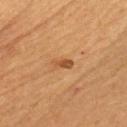The lesion was photographed on a routine skin check and not biopsied; there is no pathology result.
About 2.5 mm across.
The tile uses cross-polarized illumination.
A close-up tile cropped from a whole-body skin photograph, about 15 mm across.
The total-body-photography lesion software estimated a footprint of about 2.5 mm², a shape eccentricity near 0.9, and a symmetry-axis asymmetry near 0.35. And it measured a lesion color around L≈45 a*≈22 b*≈35 in CIELAB, roughly 10 lightness units darker than nearby skin, and a lesion-to-skin contrast of about 7.5 (normalized; higher = more distinct). The analysis additionally found a border-irregularity rating of about 3.5/10, a within-lesion color-variation index near 0.5/10, and peripheral color asymmetry of about 0. And it measured a classifier nevus-likeness of about 60/100 and a lesion-detection confidence of about 100/100.
A male patient in their mid- to late 50s.
From the chest.